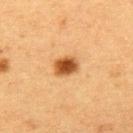Captured during whole-body skin photography for melanoma surveillance; the lesion was not biopsied. From the upper back. A lesion tile, about 15 mm wide, cut from a 3D total-body photograph. The lesion's longest dimension is about 3 mm. A female subject, aged around 40.